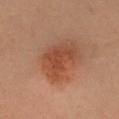No biopsy was performed on this lesion — it was imaged during a full skin examination and was not determined to be concerning. The patient is a female aged approximately 40. A region of skin cropped from a whole-body photographic capture, roughly 15 mm wide. The lesion is on the head or neck.